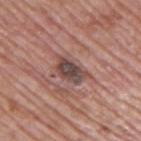follow-up: catalogued during a skin exam; not biopsied | tile lighting: white-light illumination | lesion size: ≈4.5 mm | subject: male, aged 68 to 72 | image source: total-body-photography crop, ~15 mm field of view | site: the upper back.